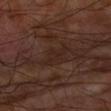The lesion was tiled from a total-body skin photograph and was not biopsied. On the arm. Automated image analysis of the tile measured a mean CIELAB color near L≈22 a*≈16 b*≈21, roughly 4 lightness units darker than nearby skin, and a normalized lesion–skin contrast near 5. And it measured a nevus-likeness score of about 0/100 and a detector confidence of about 70 out of 100 that the crop contains a lesion. This is a cross-polarized tile. This image is a 15 mm lesion crop taken from a total-body photograph. A male patient approximately 60 years of age. The recorded lesion diameter is about 2.5 mm.